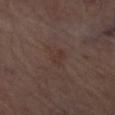| feature | finding |
|---|---|
| follow-up | catalogued during a skin exam; not biopsied |
| imaging modality | ~15 mm crop, total-body skin-cancer survey |
| TBP lesion metrics | an area of roughly 4.5 mm² and a shape-asymmetry score of about 0.2 (0 = symmetric); a mean CIELAB color near L≈34 a*≈15 b*≈21, about 4 CIELAB-L* units darker than the surrounding skin, and a normalized lesion–skin contrast near 4.5; a border-irregularity rating of about 2/10, a within-lesion color-variation index near 1.5/10, and peripheral color asymmetry of about 0.5; an automated nevus-likeness rating near 0 out of 100 and a detector confidence of about 100 out of 100 that the crop contains a lesion |
| illumination | white-light illumination |
| lesion diameter | ~2.5 mm (longest diameter) |
| body site | the right thigh |
| patient | male, in their 70s |